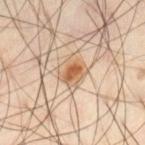<record>
<biopsy_status>not biopsied; imaged during a skin examination</biopsy_status>
<image>
  <source>total-body photography crop</source>
  <field_of_view_mm>15</field_of_view_mm>
</image>
<lesion_size>
  <long_diameter_mm_approx>3.0</long_diameter_mm_approx>
</lesion_size>
<site>left thigh</site>
<automated_metrics>
  <vs_skin_darker_L>11.0</vs_skin_darker_L>
  <vs_skin_contrast_norm>8.0</vs_skin_contrast_norm>
  <nevus_likeness_0_100>95</nevus_likeness_0_100>
  <lesion_detection_confidence_0_100>100</lesion_detection_confidence_0_100>
</automated_metrics>
<patient>
  <sex>male</sex>
  <age_approx>55</age_approx>
</patient>
</record>